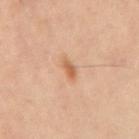diameter: ~2.5 mm (longest diameter); tile lighting: cross-polarized; anatomic site: the mid back; subject: male, approximately 50 years of age; acquisition: 15 mm crop, total-body photography.